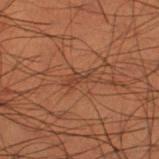biopsy status: total-body-photography surveillance lesion; no biopsy
image: ~15 mm tile from a whole-body skin photo
lesion diameter: ≈3.5 mm
automated lesion analysis: a lesion–skin lightness drop of about 5 and a normalized border contrast of about 5
site: the left thigh
patient: male, approximately 50 years of age